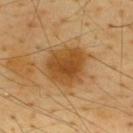No biopsy was performed on this lesion — it was imaged during a full skin examination and was not determined to be concerning. Imaged with cross-polarized lighting. A lesion tile, about 15 mm wide, cut from a 3D total-body photograph. Measured at roughly 5 mm in maximum diameter. The lesion is on the upper back. Automated image analysis of the tile measured border irregularity of about 2 on a 0–10 scale and radial color variation of about 1. It also reported an automated nevus-likeness rating near 90 out of 100 and a detector confidence of about 100 out of 100 that the crop contains a lesion. A male patient, in their mid- to late 60s.